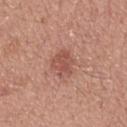No biopsy was performed on this lesion — it was imaged during a full skin examination and was not determined to be concerning. The lesion-visualizer software estimated an area of roughly 7.5 mm² and an outline eccentricity of about 0.7 (0 = round, 1 = elongated). The patient is a male about 20 years old. Located on the upper back. Approximately 3.5 mm at its widest. A roughly 15 mm field-of-view crop from a total-body skin photograph.